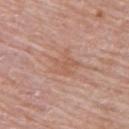The lesion was photographed on a routine skin check and not biopsied; there is no pathology result.
A region of skin cropped from a whole-body photographic capture, roughly 15 mm wide.
The patient is a female roughly 65 years of age.
The lesion's longest dimension is about 4 mm.
Located on the back.
Imaged with white-light lighting.